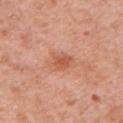Assessment:
This lesion was catalogued during total-body skin photography and was not selected for biopsy.
Clinical summary:
Located on the left upper arm. Captured under white-light illumination. An algorithmic analysis of the crop reported a lesion area of about 6 mm² and a shape-asymmetry score of about 0.25 (0 = symmetric). The analysis additionally found a mean CIELAB color near L≈58 a*≈27 b*≈35. And it measured a border-irregularity index near 2.5/10, a within-lesion color-variation index near 3/10, and peripheral color asymmetry of about 1. A close-up tile cropped from a whole-body skin photograph, about 15 mm across. The subject is a female aged approximately 40.